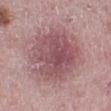biopsy status: total-body-photography surveillance lesion; no biopsy | lesion size: ≈7 mm | subject: female, about 45 years old | location: the left lower leg | tile lighting: white-light illumination | image source: ~15 mm tile from a whole-body skin photo.